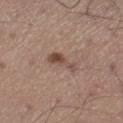workup — total-body-photography surveillance lesion; no biopsy
size — ~3.5 mm (longest diameter)
subject — male, in their mid-50s
location — the left thigh
acquisition — total-body-photography crop, ~15 mm field of view
tile lighting — white-light
automated metrics — a border-irregularity index near 5.5/10, a within-lesion color-variation index near 2/10, and radial color variation of about 0.5; lesion-presence confidence of about 100/100